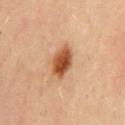follow-up = total-body-photography surveillance lesion; no biopsy | site = the mid back | image source = ~15 mm tile from a whole-body skin photo | size = about 4 mm | patient = male, aged around 50 | lighting = cross-polarized illumination.